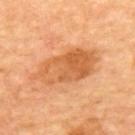– follow-up: imaged on a skin check; not biopsied
– imaging modality: ~15 mm crop, total-body skin-cancer survey
– lesion diameter: ≈7.5 mm
– patient: aged approximately 65
– automated metrics: an area of roughly 21 mm², an eccentricity of roughly 0.85, and two-axis asymmetry of about 0.15; a lesion color around L≈60 a*≈27 b*≈43 in CIELAB and a normalized lesion–skin contrast near 7; a border-irregularity rating of about 2.5/10, a color-variation rating of about 6/10, and peripheral color asymmetry of about 2; an automated nevus-likeness rating near 55 out of 100 and a detector confidence of about 100 out of 100 that the crop contains a lesion
– tile lighting: cross-polarized
– anatomic site: the back